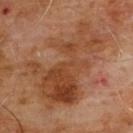workup = catalogued during a skin exam; not biopsied
subject = male, aged 58–62
image source = ~15 mm crop, total-body skin-cancer survey
site = the front of the torso